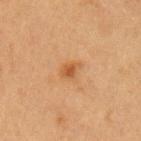| feature | finding |
|---|---|
| follow-up | catalogued during a skin exam; not biopsied |
| subject | female, in their 40s |
| location | the left upper arm |
| image | ~15 mm crop, total-body skin-cancer survey |
| lesion size | ~2.5 mm (longest diameter) |
| tile lighting | cross-polarized illumination |
| TBP lesion metrics | roughly 8 lightness units darker than nearby skin and a lesion-to-skin contrast of about 6.5 (normalized; higher = more distinct); a border-irregularity rating of about 2.5/10 and a within-lesion color-variation index near 2.5/10 |